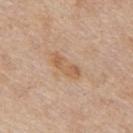A male patient in their mid- to late 50s.
A lesion tile, about 15 mm wide, cut from a 3D total-body photograph.
Captured under white-light illumination.
Automated tile analysis of the lesion measured border irregularity of about 4 on a 0–10 scale, internal color variation of about 2.5 on a 0–10 scale, and a peripheral color-asymmetry measure near 1. The analysis additionally found a classifier nevus-likeness of about 0/100 and a lesion-detection confidence of about 100/100.
About 4 mm across.
Located on the mid back.